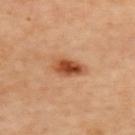Imaged during a routine full-body skin examination; the lesion was not biopsied and no histopathology is available. Measured at roughly 4 mm in maximum diameter. Automated tile analysis of the lesion measured a shape eccentricity near 0.85 and a symmetry-axis asymmetry near 0.25. A close-up tile cropped from a whole-body skin photograph, about 15 mm across. Imaged with cross-polarized lighting. On the back.